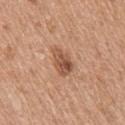{"biopsy_status": "not biopsied; imaged during a skin examination", "patient": {"sex": "female", "age_approx": 50}, "lesion_size": {"long_diameter_mm_approx": 3.5}, "image": {"source": "total-body photography crop", "field_of_view_mm": 15}, "lighting": "white-light", "site": "right upper arm"}